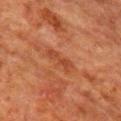<case>
<biopsy_status>not biopsied; imaged during a skin examination</biopsy_status>
<site>right upper arm</site>
<automated_metrics>
  <eccentricity>0.95</eccentricity>
  <cielab_L>36</cielab_L>
  <cielab_a>25</cielab_a>
  <cielab_b>31</cielab_b>
  <vs_skin_contrast_norm>6.0</vs_skin_contrast_norm>
  <border_irregularity_0_10>5.0</border_irregularity_0_10>
  <peripheral_color_asymmetry>0.0</peripheral_color_asymmetry>
  <lesion_detection_confidence_0_100>100</lesion_detection_confidence_0_100>
</automated_metrics>
<lighting>cross-polarized</lighting>
<image>
  <source>total-body photography crop</source>
  <field_of_view_mm>15</field_of_view_mm>
</image>
<patient>
  <sex>male</sex>
  <age_approx>80</age_approx>
</patient>
<lesion_size>
  <long_diameter_mm_approx>3.5</long_diameter_mm_approx>
</lesion_size>
</case>